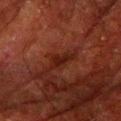patient:
  sex: male
  age_approx: 80
automated_metrics:
  cielab_L: 17
  cielab_a: 22
  cielab_b: 21
  vs_skin_darker_L: 6.0
site: right upper arm
image:
  source: total-body photography crop
  field_of_view_mm: 15
lesion_size:
  long_diameter_mm_approx: 3.0